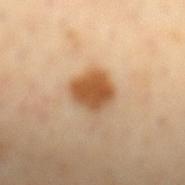Q: Was a biopsy performed?
A: total-body-photography surveillance lesion; no biopsy
Q: What kind of image is this?
A: ~15 mm crop, total-body skin-cancer survey
Q: How large is the lesion?
A: about 3.5 mm
Q: Where on the body is the lesion?
A: the mid back
Q: Patient demographics?
A: male, in their mid- to late 60s